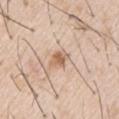{
  "biopsy_status": "not biopsied; imaged during a skin examination",
  "lighting": "white-light",
  "image": {
    "source": "total-body photography crop",
    "field_of_view_mm": 15
  },
  "patient": {
    "sex": "male",
    "age_approx": 55
  },
  "automated_metrics": {
    "cielab_L": 63,
    "cielab_a": 18,
    "cielab_b": 31,
    "color_variation_0_10": 3.5,
    "peripheral_color_asymmetry": 1.0,
    "nevus_likeness_0_100": 60,
    "lesion_detection_confidence_0_100": 100
  },
  "lesion_size": {
    "long_diameter_mm_approx": 3.0
  }
}